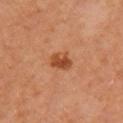Part of a total-body skin-imaging series; this lesion was reviewed on a skin check and was not flagged for biopsy. The lesion is located on the arm. Captured under cross-polarized illumination. A 15 mm close-up extracted from a 3D total-body photography capture. The subject is a female in their 60s.